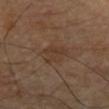Recorded during total-body skin imaging; not selected for excision or biopsy.
The lesion is on the left upper arm.
This image is a 15 mm lesion crop taken from a total-body photograph.
Captured under cross-polarized illumination.
A male subject approximately 60 years of age.
The recorded lesion diameter is about 4 mm.